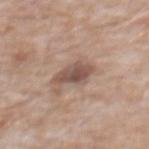The lesion was tiled from a total-body skin photograph and was not biopsied. A roughly 15 mm field-of-view crop from a total-body skin photograph. Longest diameter approximately 4 mm. Located on the chest. An algorithmic analysis of the crop reported border irregularity of about 2.5 on a 0–10 scale and a peripheral color-asymmetry measure near 1. This is a white-light tile. The subject is a male aged around 60.